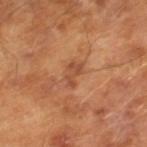The lesion was photographed on a routine skin check and not biopsied; there is no pathology result.